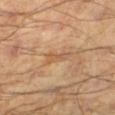A male subject aged around 45. The lesion is on the left lower leg. A region of skin cropped from a whole-body photographic capture, roughly 15 mm wide. Measured at roughly 3 mm in maximum diameter. Captured under cross-polarized illumination.